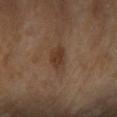This lesion was catalogued during total-body skin photography and was not selected for biopsy.
The tile uses cross-polarized illumination.
A female subject in their mid-60s.
The lesion-visualizer software estimated a border-irregularity rating of about 2/10, internal color variation of about 2.5 on a 0–10 scale, and radial color variation of about 1.
A 15 mm close-up tile from a total-body photography series done for melanoma screening.
From the right upper arm.
The lesion's longest dimension is about 3.5 mm.